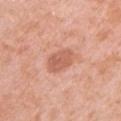Impression: No biopsy was performed on this lesion — it was imaged during a full skin examination and was not determined to be concerning. Context: A female patient, about 40 years old. A region of skin cropped from a whole-body photographic capture, roughly 15 mm wide. From the right upper arm.